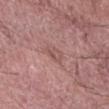Clinical impression: Captured during whole-body skin photography for melanoma surveillance; the lesion was not biopsied. Acquisition and patient details: A male patient aged around 60. The lesion is located on the right forearm. A 15 mm close-up extracted from a 3D total-body photography capture. This is a white-light tile.